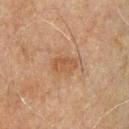  biopsy_status: not biopsied; imaged during a skin examination
  patient:
    sex: male
    age_approx: 70
  site: chest
  automated_metrics:
    shape_asymmetry: 0.25
    cielab_L: 43
    cielab_a: 17
    cielab_b: 30
    vs_skin_contrast_norm: 6.0
    border_irregularity_0_10: 3.0
    color_variation_0_10: 1.5
    peripheral_color_asymmetry: 0.5
    nevus_likeness_0_100: 0
  lesion_size:
    long_diameter_mm_approx: 3.0
  lighting: cross-polarized
  image:
    source: total-body photography crop
    field_of_view_mm: 15